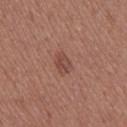Impression: Recorded during total-body skin imaging; not selected for excision or biopsy. Clinical summary: Automated tile analysis of the lesion measured a lesion area of about 4 mm², an eccentricity of roughly 0.8, and a symmetry-axis asymmetry near 0.2. It also reported a lesion color around L≈46 a*≈23 b*≈25 in CIELAB, a lesion–skin lightness drop of about 8, and a normalized border contrast of about 6. The software also gave a color-variation rating of about 3/10 and a peripheral color-asymmetry measure near 1. The analysis additionally found a classifier nevus-likeness of about 30/100 and lesion-presence confidence of about 100/100. Measured at roughly 3 mm in maximum diameter. Located on the right thigh. This image is a 15 mm lesion crop taken from a total-body photograph. The subject is a female about 40 years old.